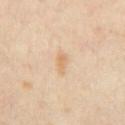Findings:
– location: the chest
– image-analysis metrics: border irregularity of about 3 on a 0–10 scale, a color-variation rating of about 2/10, and a peripheral color-asymmetry measure near 1; a classifier nevus-likeness of about 0/100 and lesion-presence confidence of about 100/100
– subject: aged 53–57
– tile lighting: cross-polarized
– acquisition: ~15 mm crop, total-body skin-cancer survey
– diameter: ≈3 mm